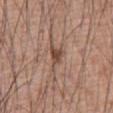Q: Is there a histopathology result?
A: total-body-photography surveillance lesion; no biopsy
Q: What kind of image is this?
A: ~15 mm tile from a whole-body skin photo
Q: Lesion size?
A: about 3.5 mm
Q: How was the tile lit?
A: white-light
Q: What is the anatomic site?
A: the abdomen
Q: What are the patient's age and sex?
A: male, about 60 years old
Q: Automated lesion metrics?
A: an average lesion color of about L≈48 a*≈19 b*≈26 (CIELAB) and roughly 10 lightness units darker than nearby skin